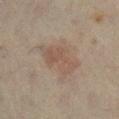The lesion was tiled from a total-body skin photograph and was not biopsied. The lesion is located on the leg. The patient is a female aged around 65. The total-body-photography lesion software estimated a lesion area of about 13 mm², a shape eccentricity near 0.85, and a shape-asymmetry score of about 0.35 (0 = symmetric). It also reported an average lesion color of about L≈44 a*≈13 b*≈23 (CIELAB) and a normalized border contrast of about 5.5. The lesion's longest dimension is about 5.5 mm. Captured under cross-polarized illumination. A 15 mm close-up extracted from a 3D total-body photography capture.